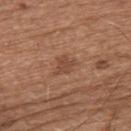Part of a total-body skin-imaging series; this lesion was reviewed on a skin check and was not flagged for biopsy.
The recorded lesion diameter is about 3 mm.
A close-up tile cropped from a whole-body skin photograph, about 15 mm across.
Imaged with white-light lighting.
A male patient aged around 75.
An algorithmic analysis of the crop reported an area of roughly 4.5 mm², an eccentricity of roughly 0.75, and a symmetry-axis asymmetry near 0.45. And it measured an automated nevus-likeness rating near 0 out of 100 and lesion-presence confidence of about 100/100.
From the upper back.